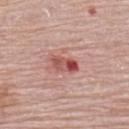workup — catalogued during a skin exam; not biopsied
body site — the upper back
TBP lesion metrics — a lesion-detection confidence of about 100/100
subject — female, approximately 65 years of age
lighting — white-light illumination
acquisition — ~15 mm tile from a whole-body skin photo